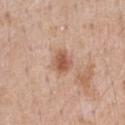| field | value |
|---|---|
| follow-up | catalogued during a skin exam; not biopsied |
| body site | the chest |
| patient | male, approximately 55 years of age |
| image | ~15 mm tile from a whole-body skin photo |
| illumination | white-light illumination |
| diameter | ≈2.5 mm |